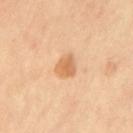Captured during whole-body skin photography for melanoma surveillance; the lesion was not biopsied. A female subject aged around 50. The lesion is located on the left thigh. Cropped from a total-body skin-imaging series; the visible field is about 15 mm.